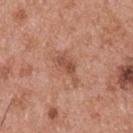biopsy status: imaged on a skin check; not biopsied | automated lesion analysis: a mean CIELAB color near L≈52 a*≈23 b*≈31 and about 9 CIELAB-L* units darker than the surrounding skin; a border-irregularity index near 5/10 and a color-variation rating of about 3/10 | lesion diameter: ≈4 mm | imaging modality: ~15 mm crop, total-body skin-cancer survey | patient: male, in their mid-50s | lighting: white-light illumination | site: the upper back.